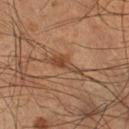Recorded during total-body skin imaging; not selected for excision or biopsy.
A 15 mm close-up tile from a total-body photography series done for melanoma screening.
This is a cross-polarized tile.
A male subject about 55 years old.
On the leg.
Longest diameter approximately 4.5 mm.